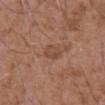Captured during whole-body skin photography for melanoma surveillance; the lesion was not biopsied.
The recorded lesion diameter is about 2.5 mm.
A 15 mm close-up extracted from a 3D total-body photography capture.
A male subject, aged 73 to 77.
From the abdomen.
The tile uses white-light illumination.